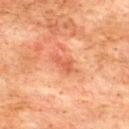Case summary:
- notes — imaged on a skin check; not biopsied
- size — ≈3 mm
- acquisition — 15 mm crop, total-body photography
- automated metrics — a symmetry-axis asymmetry near 0.3; a lesion-detection confidence of about 100/100
- subject — male, in their mid-70s
- tile lighting — cross-polarized
- location — the upper back